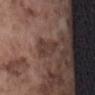Part of a total-body skin-imaging series; this lesion was reviewed on a skin check and was not flagged for biopsy. Captured under white-light illumination. A male patient aged around 75. The lesion-visualizer software estimated a lesion–skin lightness drop of about 7. The analysis additionally found a classifier nevus-likeness of about 5/100 and lesion-presence confidence of about 85/100. A region of skin cropped from a whole-body photographic capture, roughly 15 mm wide. The lesion is located on the abdomen. Longest diameter approximately 4 mm.